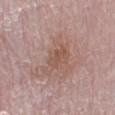{
  "biopsy_status": "not biopsied; imaged during a skin examination"
}